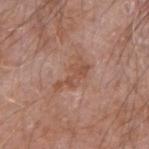The recorded lesion diameter is about 4.5 mm. The patient is a male about 60 years old. The tile uses white-light illumination. The lesion is on the right upper arm. A 15 mm close-up extracted from a 3D total-body photography capture.